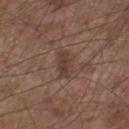An algorithmic analysis of the crop reported an area of roughly 5 mm², an eccentricity of roughly 0.85, and two-axis asymmetry of about 0.2. The software also gave a border-irregularity rating of about 2/10, a color-variation rating of about 2/10, and radial color variation of about 1. This image is a 15 mm lesion crop taken from a total-body photograph. About 3 mm across. Located on the right lower leg. A male subject, in their mid- to late 50s.